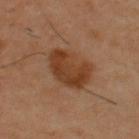The lesion was photographed on a routine skin check and not biopsied; there is no pathology result. The recorded lesion diameter is about 5 mm. The lesion is on the upper back. The tile uses cross-polarized illumination. The lesion-visualizer software estimated an eccentricity of roughly 0.7 and a symmetry-axis asymmetry near 0.2. It also reported roughly 10 lightness units darker than nearby skin and a normalized lesion–skin contrast near 9. The analysis additionally found a border-irregularity rating of about 2.5/10, internal color variation of about 3.5 on a 0–10 scale, and a peripheral color-asymmetry measure near 1. The patient is a male roughly 40 years of age. Cropped from a total-body skin-imaging series; the visible field is about 15 mm.